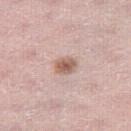| feature | finding |
|---|---|
| workup | total-body-photography surveillance lesion; no biopsy |
| patient | female, aged approximately 40 |
| acquisition | 15 mm crop, total-body photography |
| body site | the right thigh |
| tile lighting | white-light |